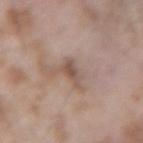Recorded during total-body skin imaging; not selected for excision or biopsy. A male subject aged 58–62. The lesion is located on the left forearm. This image is a 15 mm lesion crop taken from a total-body photograph. The total-body-photography lesion software estimated a lesion area of about 3 mm², an eccentricity of roughly 0.7, and two-axis asymmetry of about 0.45. It also reported border irregularity of about 4.5 on a 0–10 scale, a color-variation rating of about 2/10, and peripheral color asymmetry of about 0.5. The software also gave a classifier nevus-likeness of about 0/100 and a lesion-detection confidence of about 100/100. Imaged with white-light lighting.